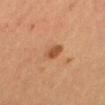No biopsy was performed on this lesion — it was imaged during a full skin examination and was not determined to be concerning. Cropped from a whole-body photographic skin survey; the tile spans about 15 mm. Located on the head or neck. The tile uses cross-polarized illumination. The total-body-photography lesion software estimated a footprint of about 5.5 mm², an eccentricity of roughly 0.75, and a symmetry-axis asymmetry near 0.15. The analysis additionally found a nevus-likeness score of about 65/100 and a lesion-detection confidence of about 100/100. The patient is a male aged around 35.